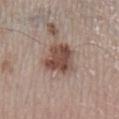Impression: Imaged during a routine full-body skin examination; the lesion was not biopsied and no histopathology is available. Context: The patient is a male about 60 years old. The recorded lesion diameter is about 5 mm. The tile uses white-light illumination. Located on the left lower leg. The lesion-visualizer software estimated an area of roughly 13 mm² and a symmetry-axis asymmetry near 0.25. A 15 mm close-up tile from a total-body photography series done for melanoma screening.